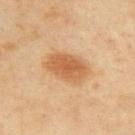biopsy_status: not biopsied; imaged during a skin examination
patient:
  sex: female
  age_approx: 60
site: upper back
lighting: cross-polarized
image:
  source: total-body photography crop
  field_of_view_mm: 15
automated_metrics:
  color_variation_0_10: 3.0
  peripheral_color_asymmetry: 1.0